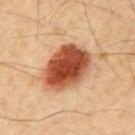{"biopsy_status": "not biopsied; imaged during a skin examination", "lighting": "cross-polarized", "site": "front of the torso", "patient": {"sex": "male", "age_approx": 60}, "image": {"source": "total-body photography crop", "field_of_view_mm": 15}}